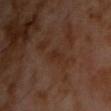notes: no biopsy performed (imaged during a skin exam) | illumination: cross-polarized | lesion diameter: ~3.5 mm (longest diameter) | automated lesion analysis: a footprint of about 3.5 mm², an eccentricity of roughly 0.9, and a symmetry-axis asymmetry near 0.3; a mean CIELAB color near L≈25 a*≈18 b*≈24, about 4 CIELAB-L* units darker than the surrounding skin, and a normalized lesion–skin contrast near 5.5; a classifier nevus-likeness of about 0/100 | acquisition: total-body-photography crop, ~15 mm field of view | subject: male, aged 58 to 62 | location: the chest.